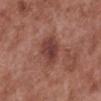notes = imaged on a skin check; not biopsied | anatomic site = the left lower leg | illumination = white-light illumination | automated lesion analysis = an area of roughly 9.5 mm², a shape eccentricity near 0.65, and two-axis asymmetry of about 0.2; a mean CIELAB color near L≈41 a*≈24 b*≈24; an automated nevus-likeness rating near 30 out of 100 and lesion-presence confidence of about 100/100 | image source = ~15 mm crop, total-body skin-cancer survey | subject = male, about 55 years old.